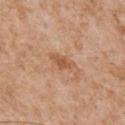Assessment: The lesion was tiled from a total-body skin photograph and was not biopsied. Background: Cropped from a whole-body photographic skin survey; the tile spans about 15 mm. Automated image analysis of the tile measured a footprint of about 4 mm² and a shape-asymmetry score of about 0.4 (0 = symmetric). This is a white-light tile. A male subject roughly 65 years of age. The lesion is located on the front of the torso.